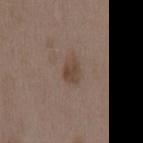biopsy_status: not biopsied; imaged during a skin examination
site: mid back
image:
  source: total-body photography crop
  field_of_view_mm: 15
patient:
  sex: female
  age_approx: 35
automated_metrics:
  area_mm2_approx: 5.0
  eccentricity: 0.7
  shape_asymmetry: 0.3
  cielab_L: 44
  cielab_a: 15
  cielab_b: 25
  vs_skin_darker_L: 8.0
  color_variation_0_10: 2.5
  peripheral_color_asymmetry: 1.0
  nevus_likeness_0_100: 50